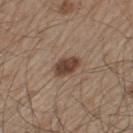About 3.5 mm across. The tile uses white-light illumination. A close-up tile cropped from a whole-body skin photograph, about 15 mm across. The patient is a male aged around 60. An algorithmic analysis of the crop reported a lesion–skin lightness drop of about 13 and a normalized lesion–skin contrast near 10. The analysis additionally found a border-irregularity rating of about 1.5/10, a color-variation rating of about 2.5/10, and peripheral color asymmetry of about 1. The analysis additionally found an automated nevus-likeness rating near 95 out of 100 and a lesion-detection confidence of about 100/100. The lesion is on the left thigh.